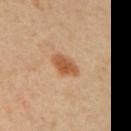Impression:
Recorded during total-body skin imaging; not selected for excision or biopsy.
Acquisition and patient details:
About 3.5 mm across. The lesion-visualizer software estimated an area of roughly 5 mm² and a symmetry-axis asymmetry near 0.25. The software also gave an automated nevus-likeness rating near 95 out of 100 and a detector confidence of about 100 out of 100 that the crop contains a lesion. A female subject aged around 65. A region of skin cropped from a whole-body photographic capture, roughly 15 mm wide. The tile uses cross-polarized illumination. The lesion is located on the mid back.